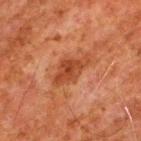subject — male, aged 78–82 | body site — the upper back | lighting — cross-polarized | acquisition — 15 mm crop, total-body photography.